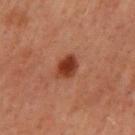Captured during whole-body skin photography for melanoma surveillance; the lesion was not biopsied. From the mid back. A 15 mm close-up extracted from a 3D total-body photography capture. The tile uses cross-polarized illumination. The subject is a male aged approximately 60. The total-body-photography lesion software estimated a footprint of about 5 mm², an outline eccentricity of about 0.45 (0 = round, 1 = elongated), and a symmetry-axis asymmetry near 0.2. And it measured border irregularity of about 1.5 on a 0–10 scale, a within-lesion color-variation index near 2/10, and radial color variation of about 0.5. The software also gave a nevus-likeness score of about 100/100 and a lesion-detection confidence of about 100/100.